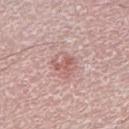Assessment: Recorded during total-body skin imaging; not selected for excision or biopsy. Context: Captured under white-light illumination. A lesion tile, about 15 mm wide, cut from a 3D total-body photograph. The patient is a male in their 50s. The recorded lesion diameter is about 2.5 mm. Located on the right lower leg.